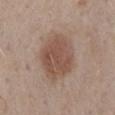- biopsy status — no biopsy performed (imaged during a skin exam)
- patient — male, approximately 55 years of age
- TBP lesion metrics — an area of roughly 19 mm², an eccentricity of roughly 0.55, and two-axis asymmetry of about 0.15; a nevus-likeness score of about 90/100
- location — the mid back
- image — total-body-photography crop, ~15 mm field of view
- lesion diameter — ≈5.5 mm
- lighting — white-light illumination